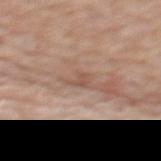The lesion was photographed on a routine skin check and not biopsied; there is no pathology result. A female patient in their mid- to late 60s. The lesion-visualizer software estimated an eccentricity of roughly 0.8. The software also gave a color-variation rating of about 1/10 and radial color variation of about 0.5. The software also gave an automated nevus-likeness rating near 0 out of 100 and lesion-presence confidence of about 80/100. The tile uses white-light illumination. Located on the upper back. Cropped from a total-body skin-imaging series; the visible field is about 15 mm.